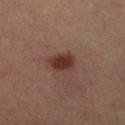Recorded during total-body skin imaging; not selected for excision or biopsy.
This image is a 15 mm lesion crop taken from a total-body photograph.
Captured under cross-polarized illumination.
Approximately 3 mm at its widest.
A female patient about 60 years old.
The lesion is located on the right lower leg.